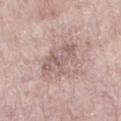notes: no biopsy performed (imaged during a skin exam)
lesion size: ~5.5 mm (longest diameter)
anatomic site: the left lower leg
image-analysis metrics: an area of roughly 14 mm², an eccentricity of roughly 0.7, and a shape-asymmetry score of about 0.3 (0 = symmetric); a detector confidence of about 100 out of 100 that the crop contains a lesion
patient: female, approximately 70 years of age
image: 15 mm crop, total-body photography